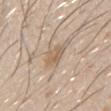This lesion was catalogued during total-body skin photography and was not selected for biopsy.
Automated tile analysis of the lesion measured a mean CIELAB color near L≈60 a*≈14 b*≈30 and about 8 CIELAB-L* units darker than the surrounding skin. It also reported a border-irregularity rating of about 2/10, a within-lesion color-variation index near 3.5/10, and a peripheral color-asymmetry measure near 1. The software also gave a classifier nevus-likeness of about 25/100 and a lesion-detection confidence of about 95/100.
Longest diameter approximately 2.5 mm.
The lesion is located on the right upper arm.
This image is a 15 mm lesion crop taken from a total-body photograph.
A male subject aged 28–32.